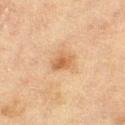Impression: The lesion was tiled from a total-body skin photograph and was not biopsied. Context: The lesion is on the right thigh. Cropped from a total-body skin-imaging series; the visible field is about 15 mm. Longest diameter approximately 2.5 mm. The subject is a female approximately 55 years of age. Automated image analysis of the tile measured a footprint of about 4.5 mm² and a shape-asymmetry score of about 0.2 (0 = symmetric). It also reported border irregularity of about 2 on a 0–10 scale and radial color variation of about 0.5. This is a cross-polarized tile.